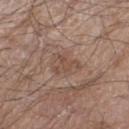Impression: No biopsy was performed on this lesion — it was imaged during a full skin examination and was not determined to be concerning. Context: On the left thigh. The tile uses white-light illumination. The lesion-visualizer software estimated a lesion color around L≈48 a*≈18 b*≈26 in CIELAB, a lesion–skin lightness drop of about 7, and a lesion-to-skin contrast of about 5.5 (normalized; higher = more distinct). And it measured a border-irregularity index near 7.5/10 and internal color variation of about 0.5 on a 0–10 scale. It also reported an automated nevus-likeness rating near 0 out of 100. A male patient approximately 80 years of age. A region of skin cropped from a whole-body photographic capture, roughly 15 mm wide.